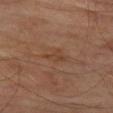<tbp_lesion>
  <biopsy_status>not biopsied; imaged during a skin examination</biopsy_status>
  <patient>
    <sex>male</sex>
    <age_approx>70</age_approx>
  </patient>
  <lesion_size>
    <long_diameter_mm_approx>3.0</long_diameter_mm_approx>
  </lesion_size>
  <lighting>cross-polarized</lighting>
  <automated_metrics>
    <area_mm2_approx>3.5</area_mm2_approx>
    <vs_skin_darker_L>5.0</vs_skin_darker_L>
    <vs_skin_contrast_norm>5.0</vs_skin_contrast_norm>
    <nevus_likeness_0_100>0</nevus_likeness_0_100>
  </automated_metrics>
  <image>
    <source>total-body photography crop</source>
    <field_of_view_mm>15</field_of_view_mm>
  </image>
  <site>left thigh</site>
</tbp_lesion>